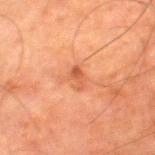image:
  source: total-body photography crop
  field_of_view_mm: 15
patient:
  sex: male
  age_approx: 80
automated_metrics:
  area_mm2_approx: 3.0
  eccentricity: 0.85
  shape_asymmetry: 0.3
  nevus_likeness_0_100: 0
  lesion_detection_confidence_0_100: 100
site: right thigh
lighting: cross-polarized